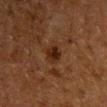follow-up: imaged on a skin check; not biopsied | lighting: cross-polarized | patient: male, aged 58–62 | site: the left upper arm | size: about 2.5 mm | acquisition: total-body-photography crop, ~15 mm field of view.